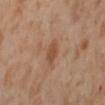Assessment: Recorded during total-body skin imaging; not selected for excision or biopsy. Context: A close-up tile cropped from a whole-body skin photograph, about 15 mm across. A female subject in their mid- to late 50s. From the back.